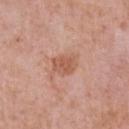Clinical impression: Captured during whole-body skin photography for melanoma surveillance; the lesion was not biopsied. Acquisition and patient details: A 15 mm crop from a total-body photograph taken for skin-cancer surveillance. The total-body-photography lesion software estimated a lesion area of about 6 mm² and two-axis asymmetry of about 0.25. The software also gave border irregularity of about 2.5 on a 0–10 scale, internal color variation of about 2.5 on a 0–10 scale, and radial color variation of about 1. A male subject, in their 50s. Located on the chest. The recorded lesion diameter is about 3 mm. The tile uses white-light illumination.